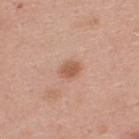Clinical impression: Recorded during total-body skin imaging; not selected for excision or biopsy.